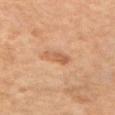Assessment:
Imaged during a routine full-body skin examination; the lesion was not biopsied and no histopathology is available.
Image and clinical context:
The lesion is located on the left upper arm. The subject is a male aged around 65. This image is a 15 mm lesion crop taken from a total-body photograph. The tile uses cross-polarized illumination.